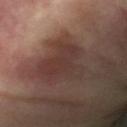| key | value |
|---|---|
| biopsy status | catalogued during a skin exam; not biopsied |
| automated metrics | a mean CIELAB color near L≈35 a*≈18 b*≈20, a lesion–skin lightness drop of about 6, and a normalized lesion–skin contrast near 5.5; border irregularity of about 6.5 on a 0–10 scale, a color-variation rating of about 3.5/10, and a peripheral color-asymmetry measure near 1.5; a detector confidence of about 80 out of 100 that the crop contains a lesion |
| site | the arm |
| image source | 15 mm crop, total-body photography |
| lesion diameter | ≈5.5 mm |
| subject | female, in their mid-70s |